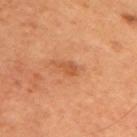Impression:
This lesion was catalogued during total-body skin photography and was not selected for biopsy.
Acquisition and patient details:
Located on the upper back. The subject is a male approximately 70 years of age. The tile uses cross-polarized illumination. Cropped from a whole-body photographic skin survey; the tile spans about 15 mm.